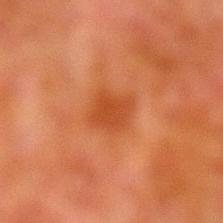The lesion was photographed on a routine skin check and not biopsied; there is no pathology result.
Automated tile analysis of the lesion measured a lesion area of about 6 mm², a shape eccentricity near 0.45, and two-axis asymmetry of about 0.2. And it measured a lesion color around L≈38 a*≈28 b*≈36 in CIELAB, roughly 7 lightness units darker than nearby skin, and a normalized border contrast of about 6.5.
A male subject, approximately 80 years of age.
On the left lower leg.
Cropped from a total-body skin-imaging series; the visible field is about 15 mm.